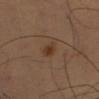Case summary:
* follow-up · imaged on a skin check; not biopsied
* tile lighting · cross-polarized
* site · the right thigh
* image · ~15 mm tile from a whole-body skin photo
* diameter · ≈3 mm
* subject · male, in their 60s